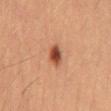Context: The lesion-visualizer software estimated a shape eccentricity near 0.8 and a shape-asymmetry score of about 0.3 (0 = symmetric). The analysis additionally found a border-irregularity rating of about 2.5/10 and a color-variation rating of about 4/10. And it measured a classifier nevus-likeness of about 100/100 and a lesion-detection confidence of about 100/100. Captured under cross-polarized illumination. A close-up tile cropped from a whole-body skin photograph, about 15 mm across. A male subject aged 53 to 57. From the abdomen. Measured at roughly 3 mm in maximum diameter.